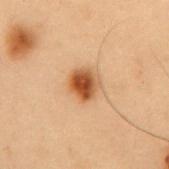{"site": "mid back", "patient": {"sex": "male", "age_approx": 55}, "image": {"source": "total-body photography crop", "field_of_view_mm": 15}, "lesion_size": {"long_diameter_mm_approx": 3.0}, "automated_metrics": {"cielab_L": 44, "cielab_a": 21, "cielab_b": 34, "vs_skin_darker_L": 13.0, "vs_skin_contrast_norm": 10.5}, "lighting": "cross-polarized"}